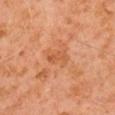Impression:
Recorded during total-body skin imaging; not selected for excision or biopsy.
Clinical summary:
This is a cross-polarized tile. This image is a 15 mm lesion crop taken from a total-body photograph. On the right upper arm. A male subject aged 58 to 62. Automated tile analysis of the lesion measured a border-irregularity index near 4/10, a within-lesion color-variation index near 0/10, and a peripheral color-asymmetry measure near 0. The lesion's longest dimension is about 3 mm.